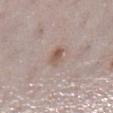workup: imaged on a skin check; not biopsied | patient: female, about 30 years old | site: the left lower leg | acquisition: 15 mm crop, total-body photography | illumination: white-light | size: about 3 mm.